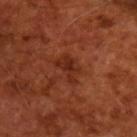biopsy status: imaged on a skin check; not biopsied | image-analysis metrics: a lesion area of about 6 mm², an eccentricity of roughly 0.8, and a symmetry-axis asymmetry near 0.4; a lesion–skin lightness drop of about 7 and a lesion-to-skin contrast of about 7 (normalized; higher = more distinct); a border-irregularity rating of about 5/10 and a within-lesion color-variation index near 2/10 | imaging modality: ~15 mm crop, total-body skin-cancer survey | subject: male, about 65 years old | illumination: cross-polarized.